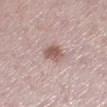Clinical impression: Recorded during total-body skin imaging; not selected for excision or biopsy. Context: A female patient in their mid-60s. Automated tile analysis of the lesion measured a footprint of about 5.5 mm², an outline eccentricity of about 0.5 (0 = round, 1 = elongated), and a symmetry-axis asymmetry near 0.25. The analysis additionally found a border-irregularity rating of about 2.5/10, internal color variation of about 3 on a 0–10 scale, and radial color variation of about 1. The tile uses white-light illumination. The lesion is on the left lower leg. Cropped from a whole-body photographic skin survey; the tile spans about 15 mm.